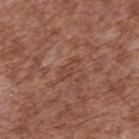Case summary:
- follow-up: imaged on a skin check; not biopsied
- subject: male, aged approximately 75
- imaging modality: ~15 mm tile from a whole-body skin photo
- tile lighting: white-light
- site: the upper back
- diameter: ~3.5 mm (longest diameter)